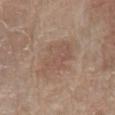<record>
  <biopsy_status>not biopsied; imaged during a skin examination</biopsy_status>
  <patient>
    <sex>female</sex>
    <age_approx>65</age_approx>
  </patient>
  <site>arm</site>
  <lighting>white-light</lighting>
  <automated_metrics>
    <border_irregularity_0_10>4.0</border_irregularity_0_10>
    <color_variation_0_10>2.5</color_variation_0_10>
    <peripheral_color_asymmetry>1.0</peripheral_color_asymmetry>
    <nevus_likeness_0_100>45</nevus_likeness_0_100>
    <lesion_detection_confidence_0_100>100</lesion_detection_confidence_0_100>
  </automated_metrics>
  <image>
    <source>total-body photography crop</source>
    <field_of_view_mm>15</field_of_view_mm>
  </image>
  <lesion_size>
    <long_diameter_mm_approx>5.0</long_diameter_mm_approx>
  </lesion_size>
</record>